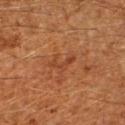Part of a total-body skin-imaging series; this lesion was reviewed on a skin check and was not flagged for biopsy.
Measured at roughly 3.5 mm in maximum diameter.
Cropped from a total-body skin-imaging series; the visible field is about 15 mm.
Captured under cross-polarized illumination.
The lesion is on the left forearm.
The subject is a male approximately 60 years of age.